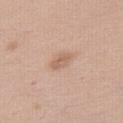biopsy status: imaged on a skin check; not biopsied
lesion diameter: about 3 mm
body site: the right thigh
imaging modality: ~15 mm tile from a whole-body skin photo
subject: female, about 40 years old